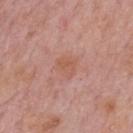Notes:
- notes · imaged on a skin check; not biopsied
- subject · male, approximately 75 years of age
- location · the mid back
- image source · 15 mm crop, total-body photography
- lesion size · ~2.5 mm (longest diameter)
- TBP lesion metrics · a lesion area of about 5 mm², a shape eccentricity near 0.45, and a shape-asymmetry score of about 0.25 (0 = symmetric)
- illumination · white-light illumination